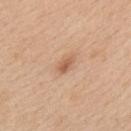| key | value |
|---|---|
| notes | catalogued during a skin exam; not biopsied |
| lesion size | ~2.5 mm (longest diameter) |
| image source | total-body-photography crop, ~15 mm field of view |
| tile lighting | white-light |
| anatomic site | the back |
| patient | male, roughly 60 years of age |